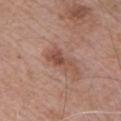Q: Is there a histopathology result?
A: imaged on a skin check; not biopsied
Q: What is the imaging modality?
A: total-body-photography crop, ~15 mm field of view
Q: Who is the patient?
A: male, in their mid-60s
Q: How was the tile lit?
A: white-light
Q: Lesion location?
A: the chest
Q: What did automated image analysis measure?
A: an area of roughly 6 mm² and two-axis asymmetry of about 0.45; roughly 9 lightness units darker than nearby skin and a normalized border contrast of about 7
Q: How large is the lesion?
A: ≈4.5 mm